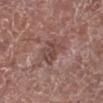TBP lesion metrics — a mean CIELAB color near L≈45 a*≈20 b*≈22 and roughly 8 lightness units darker than nearby skin; subject — male, about 75 years old; lesion diameter — about 3.5 mm; body site — the left lower leg; imaging modality — 15 mm crop, total-body photography.